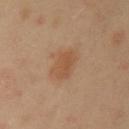Imaged during a routine full-body skin examination; the lesion was not biopsied and no histopathology is available. The lesion is located on the left upper arm. Automated image analysis of the tile measured a mean CIELAB color near L≈51 a*≈20 b*≈33, roughly 7 lightness units darker than nearby skin, and a normalized border contrast of about 6. The software also gave a nevus-likeness score of about 70/100 and a detector confidence of about 100 out of 100 that the crop contains a lesion. Cropped from a total-body skin-imaging series; the visible field is about 15 mm. A male subject, roughly 40 years of age. Longest diameter approximately 3.5 mm.